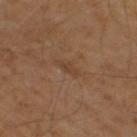Assessment:
This lesion was catalogued during total-body skin photography and was not selected for biopsy.
Context:
The lesion is on the right thigh. Imaged with cross-polarized lighting. Automated image analysis of the tile measured a lesion area of about 2.5 mm², an outline eccentricity of about 0.9 (0 = round, 1 = elongated), and two-axis asymmetry of about 0.4. And it measured a color-variation rating of about 0/10. The analysis additionally found an automated nevus-likeness rating near 0 out of 100 and a detector confidence of about 90 out of 100 that the crop contains a lesion. The patient is a male in their mid- to late 50s. Cropped from a whole-body photographic skin survey; the tile spans about 15 mm.